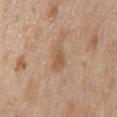The lesion was tiled from a total-body skin photograph and was not biopsied.
Longest diameter approximately 3.5 mm.
The total-body-photography lesion software estimated a footprint of about 4.5 mm² and two-axis asymmetry of about 0.3. The software also gave a mean CIELAB color near L≈56 a*≈19 b*≈33 and about 8 CIELAB-L* units darker than the surrounding skin. The analysis additionally found a border-irregularity index near 3/10. And it measured an automated nevus-likeness rating near 5 out of 100 and a detector confidence of about 100 out of 100 that the crop contains a lesion.
On the chest.
The tile uses white-light illumination.
A male patient, aged around 65.
Cropped from a whole-body photographic skin survey; the tile spans about 15 mm.